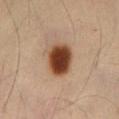• follow-up: imaged on a skin check; not biopsied
• size: about 4 mm
• acquisition: total-body-photography crop, ~15 mm field of view
• subject: male, aged 53–57
• site: the abdomen
• illumination: cross-polarized